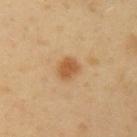Notes:
– workup: imaged on a skin check; not biopsied
– lighting: cross-polarized
– location: the left upper arm
– image source: ~15 mm tile from a whole-body skin photo
– image-analysis metrics: an area of roughly 5 mm², a shape eccentricity near 0.65, and two-axis asymmetry of about 0.15; a border-irregularity index near 1.5/10, a color-variation rating of about 2/10, and a peripheral color-asymmetry measure near 0.5
– size: ~3 mm (longest diameter)
– patient: male, aged approximately 40